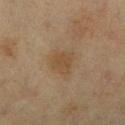follow-up: catalogued during a skin exam; not biopsied
lesion diameter: ~3 mm (longest diameter)
automated metrics: a normalized border contrast of about 6; a color-variation rating of about 1.5/10 and radial color variation of about 0.5
body site: the leg
lighting: cross-polarized illumination
subject: female, approximately 55 years of age
imaging modality: ~15 mm crop, total-body skin-cancer survey